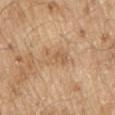Q: Was a biopsy performed?
A: imaged on a skin check; not biopsied
Q: What did automated image analysis measure?
A: an area of roughly 5.5 mm², an eccentricity of roughly 0.85, and two-axis asymmetry of about 0.4; a lesion-to-skin contrast of about 5 (normalized; higher = more distinct); border irregularity of about 5 on a 0–10 scale
Q: What are the patient's age and sex?
A: male, in their 70s
Q: What is the imaging modality?
A: total-body-photography crop, ~15 mm field of view
Q: Where on the body is the lesion?
A: the back
Q: How large is the lesion?
A: ~3.5 mm (longest diameter)